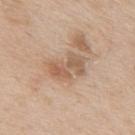The lesion was tiled from a total-body skin photograph and was not biopsied.
Located on the upper back.
The lesion's longest dimension is about 4 mm.
An algorithmic analysis of the crop reported a border-irregularity index near 5/10, internal color variation of about 5 on a 0–10 scale, and a peripheral color-asymmetry measure near 2. And it measured an automated nevus-likeness rating near 0 out of 100 and a lesion-detection confidence of about 100/100.
The subject is a male aged around 65.
A 15 mm close-up tile from a total-body photography series done for melanoma screening.